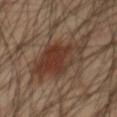The lesion was tiled from a total-body skin photograph and was not biopsied. From the chest. A male subject, aged approximately 60. Automated image analysis of the tile measured a mean CIELAB color near L≈35 a*≈18 b*≈26, about 9 CIELAB-L* units darker than the surrounding skin, and a lesion-to-skin contrast of about 8.5 (normalized; higher = more distinct). The software also gave a border-irregularity rating of about 6/10, a color-variation rating of about 4.5/10, and a peripheral color-asymmetry measure near 1.5. And it measured an automated nevus-likeness rating near 100 out of 100 and a lesion-detection confidence of about 100/100. This image is a 15 mm lesion crop taken from a total-body photograph. Captured under cross-polarized illumination.